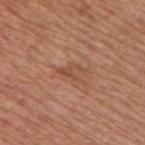| feature | finding |
|---|---|
| follow-up | total-body-photography surveillance lesion; no biopsy |
| image | ~15 mm tile from a whole-body skin photo |
| body site | the back |
| patient | male, about 65 years old |
| lesion size | ≈3 mm |
| image-analysis metrics | a lesion area of about 4 mm² and two-axis asymmetry of about 0.5; an automated nevus-likeness rating near 0 out of 100 |
| illumination | white-light illumination |